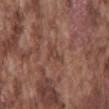This lesion was catalogued during total-body skin photography and was not selected for biopsy. This image is a 15 mm lesion crop taken from a total-body photograph. The tile uses white-light illumination. The subject is a male about 75 years old. The lesion is on the mid back.